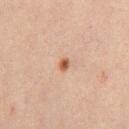• tile lighting — cross-polarized illumination
• automated metrics — a mean CIELAB color near L≈46 a*≈21 b*≈29, about 12 CIELAB-L* units darker than the surrounding skin, and a lesion-to-skin contrast of about 9.5 (normalized; higher = more distinct); a color-variation rating of about 1.5/10 and a peripheral color-asymmetry measure near 0.5
• anatomic site — the chest
• diameter — about 1.5 mm
• image source — ~15 mm crop, total-body skin-cancer survey
• patient — male, approximately 30 years of age